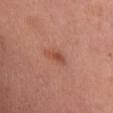  biopsy_status: not biopsied; imaged during a skin examination